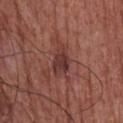The lesion was tiled from a total-body skin photograph and was not biopsied.
Longest diameter approximately 4.5 mm.
Cropped from a whole-body photographic skin survey; the tile spans about 15 mm.
Located on the chest.
The patient is a male aged 73–77.
The total-body-photography lesion software estimated an area of roughly 7.5 mm², an eccentricity of roughly 0.85, and two-axis asymmetry of about 0.25. The software also gave internal color variation of about 4 on a 0–10 scale and a peripheral color-asymmetry measure near 1.5. The software also gave an automated nevus-likeness rating near 5 out of 100 and lesion-presence confidence of about 100/100.
This is a white-light tile.